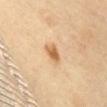biopsy_status: not biopsied; imaged during a skin examination
patient:
  sex: female
  age_approx: 60
site: chest
automated_metrics:
  vs_skin_darker_L: 13.0
  vs_skin_contrast_norm: 8.5
  border_irregularity_0_10: 2.5
  peripheral_color_asymmetry: 1.0
  nevus_likeness_0_100: 90
lesion_size:
  long_diameter_mm_approx: 2.5
image:
  source: total-body photography crop
  field_of_view_mm: 15
lighting: cross-polarized